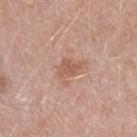Notes:
- notes: no biopsy performed (imaged during a skin exam)
- patient: female, in their mid-40s
- acquisition: 15 mm crop, total-body photography
- body site: the left forearm
- lesion diameter: about 2.5 mm